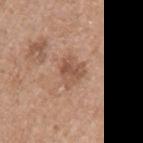Part of a total-body skin-imaging series; this lesion was reviewed on a skin check and was not flagged for biopsy. The lesion is located on the left upper arm. A female patient approximately 70 years of age. Imaged with white-light lighting. A roughly 15 mm field-of-view crop from a total-body skin photograph.